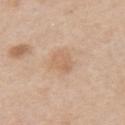site: the arm
diameter: ≈3.5 mm
image-analysis metrics: a lesion color around L≈64 a*≈18 b*≈33 in CIELAB and a normalized lesion–skin contrast near 5; border irregularity of about 2 on a 0–10 scale and a color-variation rating of about 3/10; a classifier nevus-likeness of about 10/100 and a detector confidence of about 100 out of 100 that the crop contains a lesion
illumination: white-light illumination
image: ~15 mm tile from a whole-body skin photo
patient: male, approximately 60 years of age